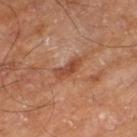  patient:
    sex: male
    age_approx: 65
  image:
    source: total-body photography crop
    field_of_view_mm: 15
  site: left thigh
  lighting: cross-polarized
  lesion_size:
    long_diameter_mm_approx: 3.5